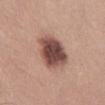This lesion was catalogued during total-body skin photography and was not selected for biopsy. A male subject, in their mid- to late 20s. The lesion is on the abdomen. A region of skin cropped from a whole-body photographic capture, roughly 15 mm wide.